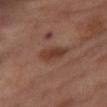Notes:
– follow-up: no biopsy performed (imaged during a skin exam)
– lesion diameter: ~4 mm (longest diameter)
– subject: female, about 55 years old
– image source: total-body-photography crop, ~15 mm field of view
– site: the front of the torso
– image-analysis metrics: a footprint of about 6.5 mm², an outline eccentricity of about 0.85 (0 = round, 1 = elongated), and two-axis asymmetry of about 0.25; an average lesion color of about L≈39 a*≈21 b*≈27 (CIELAB), about 9 CIELAB-L* units darker than the surrounding skin, and a normalized border contrast of about 7.5; internal color variation of about 2.5 on a 0–10 scale and peripheral color asymmetry of about 0.5